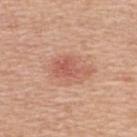lesion_size:
  long_diameter_mm_approx: 4.0
image:
  source: total-body photography crop
  field_of_view_mm: 15
patient:
  sex: female
  age_approx: 50
site: upper back